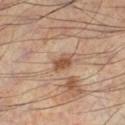The lesion was tiled from a total-body skin photograph and was not biopsied.
A roughly 15 mm field-of-view crop from a total-body skin photograph.
Longest diameter approximately 2.5 mm.
The lesion-visualizer software estimated a footprint of about 4 mm² and an eccentricity of roughly 0.75. And it measured a border-irregularity rating of about 2/10, a within-lesion color-variation index near 2/10, and peripheral color asymmetry of about 0.5. The software also gave a detector confidence of about 100 out of 100 that the crop contains a lesion.
The lesion is located on the leg.
Captured under cross-polarized illumination.
A male patient approximately 55 years of age.